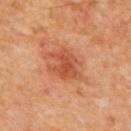Impression:
Recorded during total-body skin imaging; not selected for excision or biopsy.
Background:
A male patient, about 65 years old. This image is a 15 mm lesion crop taken from a total-body photograph. The tile uses cross-polarized illumination. Longest diameter approximately 4.5 mm. Located on the mid back.